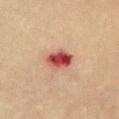No biopsy was performed on this lesion — it was imaged during a full skin examination and was not determined to be concerning. The subject is a male aged around 75. This image is a 15 mm lesion crop taken from a total-body photograph. The lesion is on the chest.